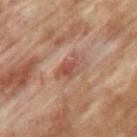biopsy status: imaged on a skin check; not biopsied
automated metrics: a footprint of about 4.5 mm², an outline eccentricity of about 0.8 (0 = round, 1 = elongated), and two-axis asymmetry of about 0.45; a mean CIELAB color near L≈48 a*≈23 b*≈27
patient: male, roughly 65 years of age
acquisition: total-body-photography crop, ~15 mm field of view
illumination: cross-polarized
body site: the left upper arm